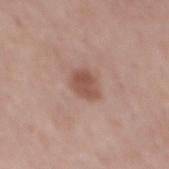Q: Was this lesion biopsied?
A: catalogued during a skin exam; not biopsied
Q: What is the lesion's diameter?
A: ~3.5 mm (longest diameter)
Q: Who is the patient?
A: male, approximately 60 years of age
Q: What kind of image is this?
A: total-body-photography crop, ~15 mm field of view
Q: Lesion location?
A: the back